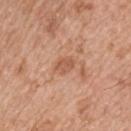workup: imaged on a skin check; not biopsied
imaging modality: ~15 mm crop, total-body skin-cancer survey
body site: the upper back
automated lesion analysis: a lesion color around L≈56 a*≈24 b*≈33 in CIELAB, a lesion–skin lightness drop of about 9, and a lesion-to-skin contrast of about 6 (normalized; higher = more distinct); border irregularity of about 2 on a 0–10 scale, internal color variation of about 2 on a 0–10 scale, and radial color variation of about 0.5
diameter: ~2.5 mm (longest diameter)
illumination: white-light illumination
subject: male, in their mid- to late 70s